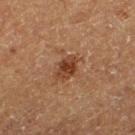Recorded during total-body skin imaging; not selected for excision or biopsy.
The lesion is located on the left thigh.
The patient is a male aged 73 to 77.
A close-up tile cropped from a whole-body skin photograph, about 15 mm across.